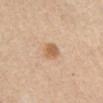biopsy status — imaged on a skin check; not biopsied | location — the abdomen | subject — female, about 65 years old | image source — ~15 mm crop, total-body skin-cancer survey.